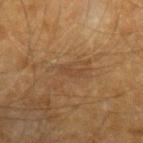<record>
<biopsy_status>not biopsied; imaged during a skin examination</biopsy_status>
<patient>
  <sex>male</sex>
  <age_approx>60</age_approx>
</patient>
<image>
  <source>total-body photography crop</source>
  <field_of_view_mm>15</field_of_view_mm>
</image>
<lighting>cross-polarized</lighting>
<site>left forearm</site>
<automated_metrics>
  <cielab_L>40</cielab_L>
  <cielab_a>18</cielab_a>
  <cielab_b>29</cielab_b>
  <vs_skin_darker_L>5.0</vs_skin_darker_L>
  <vs_skin_contrast_norm>5.0</vs_skin_contrast_norm>
  <color_variation_0_10>0.0</color_variation_0_10>
  <peripheral_color_asymmetry>0.0</peripheral_color_asymmetry>
</automated_metrics>
<lesion_size>
  <long_diameter_mm_approx>2.5</long_diameter_mm_approx>
</lesion_size>
</record>